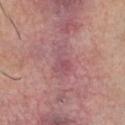notes = no biopsy performed (imaged during a skin exam)
subject = male, in their 30s
lesion diameter = ≈4 mm
imaging modality = ~15 mm crop, total-body skin-cancer survey
site = the chest